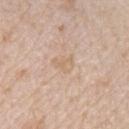Imaged during a routine full-body skin examination; the lesion was not biopsied and no histopathology is available.
The recorded lesion diameter is about 2.5 mm.
The lesion is on the chest.
The total-body-photography lesion software estimated a lesion area of about 3.5 mm² and an outline eccentricity of about 0.7 (0 = round, 1 = elongated). The software also gave a border-irregularity index near 4/10 and peripheral color asymmetry of about 0.5. It also reported an automated nevus-likeness rating near 0 out of 100 and a lesion-detection confidence of about 100/100.
The subject is a female approximately 45 years of age.
This is a white-light tile.
This image is a 15 mm lesion crop taken from a total-body photograph.